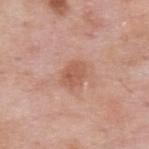The lesion was tiled from a total-body skin photograph and was not biopsied. A close-up tile cropped from a whole-body skin photograph, about 15 mm across. A male subject, roughly 55 years of age. The tile uses white-light illumination. Measured at roughly 3 mm in maximum diameter. From the upper back.